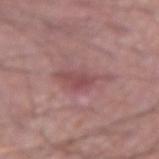{
  "image": {
    "source": "total-body photography crop",
    "field_of_view_mm": 15
  },
  "patient": {
    "sex": "male",
    "age_approx": 50
  },
  "lighting": "white-light",
  "automated_metrics": {
    "cielab_L": 49,
    "cielab_a": 24,
    "cielab_b": 19,
    "vs_skin_darker_L": 8.0,
    "vs_skin_contrast_norm": 6.0
  },
  "lesion_size": {
    "long_diameter_mm_approx": 5.0
  },
  "site": "right upper arm"
}